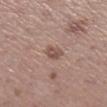Imaged during a routine full-body skin examination; the lesion was not biopsied and no histopathology is available. A female patient, aged 43–47. Cropped from a whole-body photographic skin survey; the tile spans about 15 mm. Captured under white-light illumination. Approximately 2.5 mm at its widest. On the right lower leg. Automated image analysis of the tile measured a border-irregularity index near 2.5/10 and peripheral color asymmetry of about 1. It also reported an automated nevus-likeness rating near 30 out of 100 and lesion-presence confidence of about 100/100.